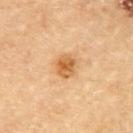workup = catalogued during a skin exam; not biopsied | image = ~15 mm crop, total-body skin-cancer survey | site = the upper back | subject = male, in their mid-80s or older.